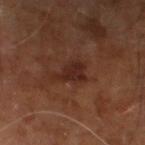biopsy_status: not biopsied; imaged during a skin examination
site: right leg
image:
  source: total-body photography crop
  field_of_view_mm: 15
lighting: cross-polarized
automated_metrics:
  cielab_L: 25
  cielab_a: 20
  cielab_b: 23
  vs_skin_contrast_norm: 8.0
patient:
  sex: male
  age_approx: 60
lesion_size:
  long_diameter_mm_approx: 4.0